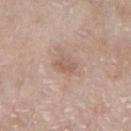follow-up: total-body-photography surveillance lesion; no biopsy | image-analysis metrics: a lesion-to-skin contrast of about 5.5 (normalized; higher = more distinct); border irregularity of about 2 on a 0–10 scale, internal color variation of about 2 on a 0–10 scale, and radial color variation of about 0.5 | site: the right lower leg | patient: female, in their mid- to late 70s | imaging modality: ~15 mm crop, total-body skin-cancer survey | diameter: ~2.5 mm (longest diameter) | tile lighting: white-light illumination.